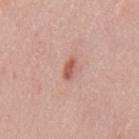Recorded during total-body skin imaging; not selected for excision or biopsy. The recorded lesion diameter is about 2.5 mm. The subject is a male aged 48 to 52. Located on the mid back. This image is a 15 mm lesion crop taken from a total-body photograph.